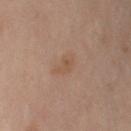{
  "biopsy_status": "not biopsied; imaged during a skin examination",
  "site": "arm",
  "image": {
    "source": "total-body photography crop",
    "field_of_view_mm": 15
  },
  "patient": {
    "sex": "female",
    "age_approx": 50
  },
  "lighting": "cross-polarized",
  "automated_metrics": {
    "area_mm2_approx": 3.5,
    "eccentricity": 0.9,
    "shape_asymmetry": 0.4,
    "border_irregularity_0_10": 4.5,
    "color_variation_0_10": 1.0,
    "peripheral_color_asymmetry": 0.5
  }
}